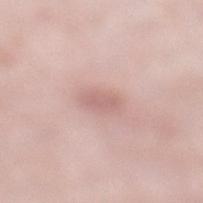Clinical impression: The lesion was photographed on a routine skin check and not biopsied; there is no pathology result. Image and clinical context: A female patient roughly 40 years of age. The lesion is located on the left lower leg. Measured at roughly 2.5 mm in maximum diameter. The tile uses white-light illumination. A 15 mm close-up extracted from a 3D total-body photography capture.